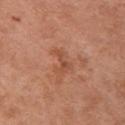{"biopsy_status": "not biopsied; imaged during a skin examination", "lesion_size": {"long_diameter_mm_approx": 3.0}, "image": {"source": "total-body photography crop", "field_of_view_mm": 15}, "patient": {"sex": "female", "age_approx": 65}, "site": "left upper arm", "lighting": "white-light"}